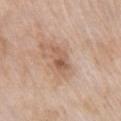| feature | finding |
|---|---|
| follow-up | catalogued during a skin exam; not biopsied |
| patient | female, aged around 75 |
| location | the front of the torso |
| tile lighting | white-light illumination |
| image | 15 mm crop, total-body photography |